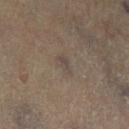Notes:
– follow-up · total-body-photography surveillance lesion; no biopsy
– location · the left lower leg
– patient · male, in their 70s
– imaging modality · 15 mm crop, total-body photography